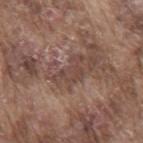{"site": "mid back", "automated_metrics": {"eccentricity": 0.8, "shape_asymmetry": 0.6, "border_irregularity_0_10": 7.5, "color_variation_0_10": 2.5, "peripheral_color_asymmetry": 1.0, "nevus_likeness_0_100": 0, "lesion_detection_confidence_0_100": 55}, "patient": {"sex": "male", "age_approx": 75}, "lighting": "white-light", "image": {"source": "total-body photography crop", "field_of_view_mm": 15}}